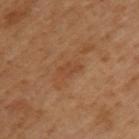biopsy_status: not biopsied; imaged during a skin examination
patient:
  sex: male
  age_approx: 50
lighting: cross-polarized
automated_metrics:
  nevus_likeness_0_100: 0
  lesion_detection_confidence_0_100: 100
image:
  source: total-body photography crop
  field_of_view_mm: 15
site: left upper arm
lesion_size:
  long_diameter_mm_approx: 3.0